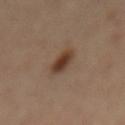follow-up: imaged on a skin check; not biopsied | lighting: cross-polarized | automated lesion analysis: an area of roughly 6 mm², an outline eccentricity of about 0.7 (0 = round, 1 = elongated), and a shape-asymmetry score of about 0.2 (0 = symmetric); an average lesion color of about L≈40 a*≈17 b*≈27 (CIELAB), about 11 CIELAB-L* units darker than the surrounding skin, and a normalized border contrast of about 9.5; a color-variation rating of about 5/10 and peripheral color asymmetry of about 1.5; a classifier nevus-likeness of about 100/100 and a lesion-detection confidence of about 100/100 | lesion diameter: ~3 mm (longest diameter) | site: the mid back | acquisition: total-body-photography crop, ~15 mm field of view.